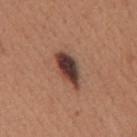workup — catalogued during a skin exam; not biopsied | location — the back | image source — 15 mm crop, total-body photography | patient — female, about 30 years old | illumination — white-light | lesion diameter — about 5 mm | automated metrics — a lesion area of about 9 mm², a shape eccentricity near 0.9, and a shape-asymmetry score of about 0.2 (0 = symmetric); border irregularity of about 2.5 on a 0–10 scale and a color-variation rating of about 7.5/10; a nevus-likeness score of about 90/100.